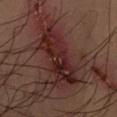This lesion was catalogued during total-body skin photography and was not selected for biopsy. The recorded lesion diameter is about 8.5 mm. The patient is a male aged approximately 40. On the right forearm. The lesion-visualizer software estimated a lesion area of about 20 mm². The analysis additionally found an average lesion color of about L≈24 a*≈22 b*≈20 (CIELAB) and a normalized border contrast of about 10. The software also gave border irregularity of about 5 on a 0–10 scale, a color-variation rating of about 6.5/10, and peripheral color asymmetry of about 2. This image is a 15 mm lesion crop taken from a total-body photograph.